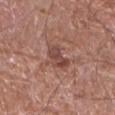Impression:
The lesion was photographed on a routine skin check and not biopsied; there is no pathology result.
Context:
An algorithmic analysis of the crop reported a lesion area of about 6 mm², an outline eccentricity of about 0.75 (0 = round, 1 = elongated), and a symmetry-axis asymmetry near 0.35. The software also gave border irregularity of about 3.5 on a 0–10 scale and radial color variation of about 1.5. The analysis additionally found a nevus-likeness score of about 0/100 and a lesion-detection confidence of about 100/100. A male subject approximately 80 years of age. Measured at roughly 3.5 mm in maximum diameter. A 15 mm crop from a total-body photograph taken for skin-cancer surveillance. Captured under white-light illumination. The lesion is on the right forearm.